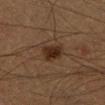Recorded during total-body skin imaging; not selected for excision or biopsy. Imaged with cross-polarized lighting. On the left lower leg. This image is a 15 mm lesion crop taken from a total-body photograph. Automated image analysis of the tile measured two-axis asymmetry of about 0.2. The analysis additionally found a nevus-likeness score of about 90/100 and a detector confidence of about 100 out of 100 that the crop contains a lesion. A male patient, approximately 40 years of age. The lesion's longest dimension is about 3 mm.